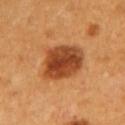- notes · imaged on a skin check; not biopsied
- lighting · cross-polarized illumination
- acquisition · ~15 mm crop, total-body skin-cancer survey
- site · the left upper arm
- diameter · ~5 mm (longest diameter)
- subject · female, approximately 50 years of age